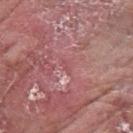This lesion was catalogued during total-body skin photography and was not selected for biopsy.
A male patient, aged around 60.
A region of skin cropped from a whole-body photographic capture, roughly 15 mm wide.
The lesion is located on the left forearm.
Automated tile analysis of the lesion measured a shape eccentricity near 0.9 and two-axis asymmetry of about 0.6. And it measured an average lesion color of about L≈51 a*≈29 b*≈19 (CIELAB), a lesion–skin lightness drop of about 5, and a normalized lesion–skin contrast near 4.